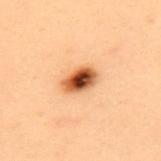Located on the upper back. Imaged with cross-polarized lighting. The patient is a female aged approximately 40. A 15 mm close-up extracted from a 3D total-body photography capture.